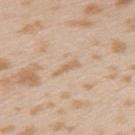Clinical impression: The lesion was photographed on a routine skin check and not biopsied; there is no pathology result. Image and clinical context: Located on the left upper arm. A female patient, roughly 25 years of age. Automated tile analysis of the lesion measured an outline eccentricity of about 0.95 (0 = round, 1 = elongated) and a symmetry-axis asymmetry near 0.4. The analysis additionally found about 7 CIELAB-L* units darker than the surrounding skin and a lesion-to-skin contrast of about 5.5 (normalized; higher = more distinct). The lesion's longest dimension is about 3 mm. A 15 mm crop from a total-body photograph taken for skin-cancer surveillance. Imaged with white-light lighting.